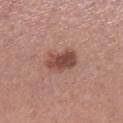Impression: The lesion was tiled from a total-body skin photograph and was not biopsied. Clinical summary: On the left lower leg. This is a white-light tile. A close-up tile cropped from a whole-body skin photograph, about 15 mm across. Longest diameter approximately 4 mm. An algorithmic analysis of the crop reported an area of roughly 8.5 mm². It also reported an automated nevus-likeness rating near 80 out of 100 and lesion-presence confidence of about 100/100. A female patient aged approximately 30.